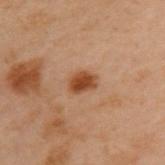Assessment:
Imaged during a routine full-body skin examination; the lesion was not biopsied and no histopathology is available.
Acquisition and patient details:
The lesion is located on the upper back. A male subject, in their mid- to late 50s. A roughly 15 mm field-of-view crop from a total-body skin photograph.